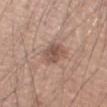image: 15 mm crop, total-body photography | location: the left forearm | tile lighting: white-light | lesion size: ≈3 mm | patient: male, about 45 years old.